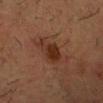<lesion>
  <biopsy_status>not biopsied; imaged during a skin examination</biopsy_status>
  <lighting>cross-polarized</lighting>
  <image>
    <source>total-body photography crop</source>
    <field_of_view_mm>15</field_of_view_mm>
  </image>
  <lesion_size>
    <long_diameter_mm_approx>6.0</long_diameter_mm_approx>
  </lesion_size>
  <site>head or neck</site>
  <patient>
    <sex>male</sex>
    <age_approx>35</age_approx>
  </patient>
</lesion>